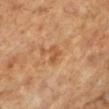Clinical impression:
Imaged during a routine full-body skin examination; the lesion was not biopsied and no histopathology is available.